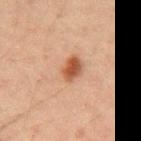Assessment:
This lesion was catalogued during total-body skin photography and was not selected for biopsy.
Acquisition and patient details:
A 15 mm close-up tile from a total-body photography series done for melanoma screening. Longest diameter approximately 6 mm. Imaged with cross-polarized lighting. The patient is a male aged around 50. The lesion is on the mid back.